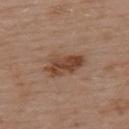Assessment:
Imaged during a routine full-body skin examination; the lesion was not biopsied and no histopathology is available.
Context:
Imaged with white-light lighting. On the upper back. The subject is a male approximately 55 years of age. A 15 mm close-up extracted from a 3D total-body photography capture. Measured at roughly 5 mm in maximum diameter.